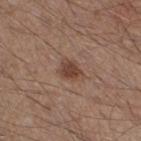Imaged during a routine full-body skin examination; the lesion was not biopsied and no histopathology is available. From the right thigh. Captured under white-light illumination. Approximately 3 mm at its widest. An algorithmic analysis of the crop reported a lesion area of about 5 mm², an outline eccentricity of about 0.65 (0 = round, 1 = elongated), and a symmetry-axis asymmetry near 0.3. The software also gave a mean CIELAB color near L≈43 a*≈19 b*≈26 and a lesion–skin lightness drop of about 10. A male patient aged around 45. Cropped from a whole-body photographic skin survey; the tile spans about 15 mm.